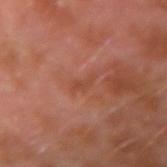No biopsy was performed on this lesion — it was imaged during a full skin examination and was not determined to be concerning. The lesion's longest dimension is about 2.5 mm. Imaged with cross-polarized lighting. A 15 mm close-up tile from a total-body photography series done for melanoma screening. The patient is a male roughly 30 years of age. From the left arm.